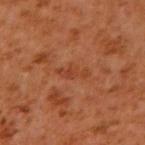biopsy status: no biopsy performed (imaged during a skin exam)
patient: male, aged 58–62
automated metrics: a footprint of about 3.5 mm² and an outline eccentricity of about 0.95 (0 = round, 1 = elongated); a lesion color around L≈39 a*≈27 b*≈34 in CIELAB, about 6 CIELAB-L* units darker than the surrounding skin, and a lesion-to-skin contrast of about 5 (normalized; higher = more distinct); a lesion-detection confidence of about 95/100
lighting: cross-polarized
image source: total-body-photography crop, ~15 mm field of view
lesion size: about 3.5 mm
location: the arm